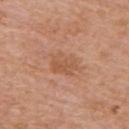Clinical impression: The lesion was tiled from a total-body skin photograph and was not biopsied. Background: The lesion is located on the upper back. The subject is a male aged around 75. Cropped from a total-body skin-imaging series; the visible field is about 15 mm. About 4 mm across.